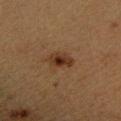A 15 mm crop from a total-body photograph taken for skin-cancer surveillance. The patient is a female aged around 40. Imaged with cross-polarized lighting. The lesion is located on the right forearm. The recorded lesion diameter is about 3 mm.